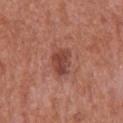Recorded during total-body skin imaging; not selected for excision or biopsy. Cropped from a total-body skin-imaging series; the visible field is about 15 mm. From the chest. The patient is a male roughly 65 years of age.